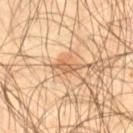| field | value |
|---|---|
| lighting | cross-polarized |
| image source | ~15 mm crop, total-body skin-cancer survey |
| subject | male, in their mid-50s |
| size | ≈4 mm |
| location | the right thigh |